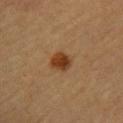biopsy_status: not biopsied; imaged during a skin examination
automated_metrics:
  area_mm2_approx: 5.5
  eccentricity: 0.5
  cielab_L: 34
  cielab_a: 19
  cielab_b: 32
  vs_skin_darker_L: 11.0
  border_irregularity_0_10: 1.5
  peripheral_color_asymmetry: 1.0
  nevus_likeness_0_100: 100
  lesion_detection_confidence_0_100: 100
lesion_size:
  long_diameter_mm_approx: 3.0
lighting: cross-polarized
site: arm
patient:
  sex: female
  age_approx: 60
image:
  source: total-body photography crop
  field_of_view_mm: 15